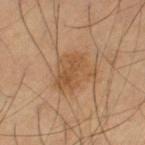The lesion was tiled from a total-body skin photograph and was not biopsied.